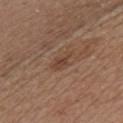The lesion was photographed on a routine skin check and not biopsied; there is no pathology result.
Imaged with white-light lighting.
The lesion is located on the chest.
Automated image analysis of the tile measured an outline eccentricity of about 0.85 (0 = round, 1 = elongated) and a symmetry-axis asymmetry near 0.35. And it measured a mean CIELAB color near L≈43 a*≈18 b*≈28 and a normalized border contrast of about 6.
The patient is a female aged 58 to 62.
Cropped from a whole-body photographic skin survey; the tile spans about 15 mm.
Longest diameter approximately 3 mm.